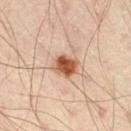No biopsy was performed on this lesion — it was imaged during a full skin examination and was not determined to be concerning.
The lesion-visualizer software estimated an automated nevus-likeness rating near 100 out of 100 and a detector confidence of about 100 out of 100 that the crop contains a lesion.
This image is a 15 mm lesion crop taken from a total-body photograph.
Longest diameter approximately 3 mm.
The patient is a male aged 38 to 42.
This is a cross-polarized tile.
The lesion is located on the leg.